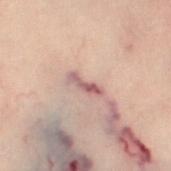The lesion was tiled from a total-body skin photograph and was not biopsied.
The recorded lesion diameter is about 3.5 mm.
Automated tile analysis of the lesion measured a footprint of about 4.5 mm², a shape eccentricity near 0.95, and a symmetry-axis asymmetry near 0.3. The analysis additionally found an average lesion color of about L≈52 a*≈19 b*≈19 (CIELAB) and a normalized border contrast of about 7.5. It also reported a border-irregularity rating of about 3.5/10, internal color variation of about 3 on a 0–10 scale, and peripheral color asymmetry of about 0.5.
The lesion is on the right leg.
The patient is a female approximately 60 years of age.
This image is a 15 mm lesion crop taken from a total-body photograph.
This is a cross-polarized tile.